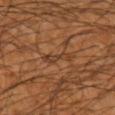The lesion was tiled from a total-body skin photograph and was not biopsied.
Approximately 3.5 mm at its widest.
The lesion is on the arm.
A 15 mm crop from a total-body photograph taken for skin-cancer surveillance.
The tile uses cross-polarized illumination.
The patient is a male aged around 60.
Automated tile analysis of the lesion measured a mean CIELAB color near L≈38 a*≈20 b*≈32, about 6 CIELAB-L* units darker than the surrounding skin, and a normalized lesion–skin contrast near 5.5.